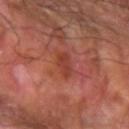patient=male, aged 58 to 62
size=~2.5 mm (longest diameter)
image source=~15 mm tile from a whole-body skin photo
site=the left forearm
tile lighting=cross-polarized